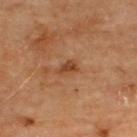biopsy status=total-body-photography surveillance lesion; no biopsy
size=~2.5 mm (longest diameter)
image=total-body-photography crop, ~15 mm field of view
body site=the upper back
illumination=cross-polarized illumination
patient=male, in their mid- to late 60s